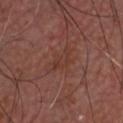| field | value |
|---|---|
| biopsy status | total-body-photography surveillance lesion; no biopsy |
| subject | male, approximately 65 years of age |
| size | ≈3 mm |
| acquisition | ~15 mm tile from a whole-body skin photo |
| site | the head or neck |
| automated metrics | a shape eccentricity near 0.8; a lesion color around L≈36 a*≈22 b*≈25 in CIELAB, roughly 4 lightness units darker than nearby skin, and a normalized border contrast of about 5; a border-irregularity index near 3/10, a color-variation rating of about 2.5/10, and peripheral color asymmetry of about 1; lesion-presence confidence of about 100/100 |
| lighting | cross-polarized |